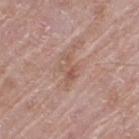Findings:
- notes: total-body-photography surveillance lesion; no biopsy
- lesion size: ≈3 mm
- image source: ~15 mm tile from a whole-body skin photo
- body site: the right thigh
- patient: male, approximately 60 years of age